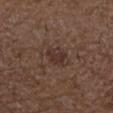Part of a total-body skin-imaging series; this lesion was reviewed on a skin check and was not flagged for biopsy.
The lesion-visualizer software estimated a footprint of about 4.5 mm², an outline eccentricity of about 0.6 (0 = round, 1 = elongated), and two-axis asymmetry of about 0.3. And it measured an average lesion color of about L≈32 a*≈17 b*≈22 (CIELAB), about 7 CIELAB-L* units darker than the surrounding skin, and a normalized lesion–skin contrast near 6.5. The analysis additionally found a lesion-detection confidence of about 100/100.
A male patient, aged 48–52.
The lesion is located on the right forearm.
This image is a 15 mm lesion crop taken from a total-body photograph.
The lesion's longest dimension is about 3 mm.
Imaged with white-light lighting.